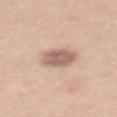  biopsy_status: not biopsied; imaged during a skin examination
  patient:
    sex: male
    age_approx: 50
  site: abdomen
  image:
    source: total-body photography crop
    field_of_view_mm: 15